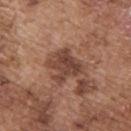Notes:
– location — the back
– patient — male, about 75 years old
– size — ~4 mm (longest diameter)
– image — ~15 mm tile from a whole-body skin photo
– tile lighting — white-light